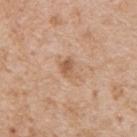site: the upper back
tile lighting: white-light
acquisition: total-body-photography crop, ~15 mm field of view
TBP lesion metrics: a mean CIELAB color near L≈58 a*≈20 b*≈33 and a normalized border contrast of about 6.5; a classifier nevus-likeness of about 0/100 and lesion-presence confidence of about 100/100
lesion diameter: ≈2.5 mm
patient: male, approximately 65 years of age